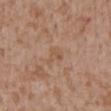• notes — no biopsy performed (imaged during a skin exam)
• location — the front of the torso
• automated metrics — a lesion area of about 3.5 mm², an outline eccentricity of about 0.75 (0 = round, 1 = elongated), and a symmetry-axis asymmetry near 0.45; an average lesion color of about L≈54 a*≈18 b*≈31 (CIELAB) and a normalized border contrast of about 4.5
• size — ≈2.5 mm
• subject — male, aged 43–47
• image — total-body-photography crop, ~15 mm field of view